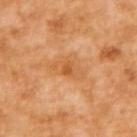  biopsy_status: not biopsied; imaged during a skin examination
  site: upper back
  image:
    source: total-body photography crop
    field_of_view_mm: 15
  patient:
    sex: female
    age_approx: 55
  lesion_size:
    long_diameter_mm_approx: 5.0